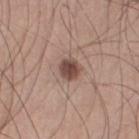Notes:
• workup · no biopsy performed (imaged during a skin exam)
• anatomic site · the left thigh
• lesion size · ~2.5 mm (longest diameter)
• subject · male, aged 28–32
• lighting · white-light
• image source · ~15 mm tile from a whole-body skin photo
• image-analysis metrics · an area of roughly 5.5 mm², an eccentricity of roughly 0.45, and a symmetry-axis asymmetry near 0.15; a border-irregularity rating of about 1.5/10, a color-variation rating of about 4/10, and a peripheral color-asymmetry measure near 1.5